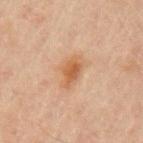Automated image analysis of the tile measured a shape eccentricity near 0.8. It also reported border irregularity of about 3 on a 0–10 scale, internal color variation of about 4 on a 0–10 scale, and peripheral color asymmetry of about 1. The recorded lesion diameter is about 4 mm. Located on the left upper arm. A male patient, in their mid- to late 40s. A 15 mm crop from a total-body photograph taken for skin-cancer surveillance. Captured under cross-polarized illumination.